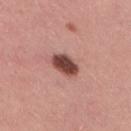{"biopsy_status": "not biopsied; imaged during a skin examination", "lighting": "white-light", "image": {"source": "total-body photography crop", "field_of_view_mm": 15}, "patient": {"sex": "female", "age_approx": 30}, "lesion_size": {"long_diameter_mm_approx": 3.5}, "site": "leg"}